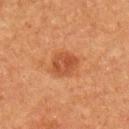Q: Was this lesion biopsied?
A: catalogued during a skin exam; not biopsied
Q: Where on the body is the lesion?
A: the left upper arm
Q: Patient demographics?
A: male, roughly 75 years of age
Q: What is the imaging modality?
A: 15 mm crop, total-body photography